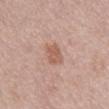Impression: Recorded during total-body skin imaging; not selected for excision or biopsy.